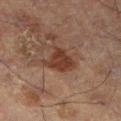* follow-up · imaged on a skin check; not biopsied
* acquisition · ~15 mm crop, total-body skin-cancer survey
* body site · the left leg
* illumination · cross-polarized illumination
* subject · male, approximately 60 years of age
* image-analysis metrics · a lesion area of about 8.5 mm², a shape eccentricity near 0.4, and a shape-asymmetry score of about 0.45 (0 = symmetric); an average lesion color of about L≈27 a*≈16 b*≈22 (CIELAB), a lesion–skin lightness drop of about 8, and a lesion-to-skin contrast of about 8.5 (normalized; higher = more distinct); a border-irregularity rating of about 4.5/10, a color-variation rating of about 2.5/10, and a peripheral color-asymmetry measure near 1
* lesion diameter · ~3.5 mm (longest diameter)